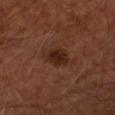Assessment: The lesion was tiled from a total-body skin photograph and was not biopsied. Acquisition and patient details: A 15 mm crop from a total-body photograph taken for skin-cancer surveillance. A male patient, roughly 65 years of age. The lesion is located on the left forearm.